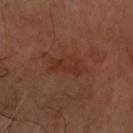Case summary:
• biopsy status — imaged on a skin check; not biopsied
• lesion size — ~4.5 mm (longest diameter)
• body site — the right forearm
• subject — aged 63 to 67
• image-analysis metrics — a nevus-likeness score of about 0/100 and lesion-presence confidence of about 100/100
• acquisition — ~15 mm tile from a whole-body skin photo